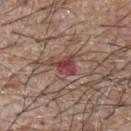biopsy_status: not biopsied; imaged during a skin examination
automated_metrics:
  cielab_L: 43
  cielab_a: 25
  cielab_b: 21
  vs_skin_contrast_norm: 9.0
  border_irregularity_0_10: 5.0
  color_variation_0_10: 4.0
  peripheral_color_asymmetry: 1.5
  nevus_likeness_0_100: 0
  lesion_detection_confidence_0_100: 100
lesion_size:
  long_diameter_mm_approx: 3.0
site: upper back
patient:
  sex: male
  age_approx: 65
image:
  source: total-body photography crop
  field_of_view_mm: 15